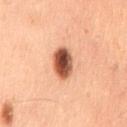The lesion was tiled from a total-body skin photograph and was not biopsied. From the lower back. A male subject about 60 years old. Imaged with cross-polarized lighting. Cropped from a total-body skin-imaging series; the visible field is about 15 mm.